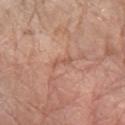{"biopsy_status": "not biopsied; imaged during a skin examination", "image": {"source": "total-body photography crop", "field_of_view_mm": 15}, "site": "left forearm", "automated_metrics": {"vs_skin_darker_L": 7.0, "vs_skin_contrast_norm": 5.0, "border_irregularity_0_10": 5.5, "nevus_likeness_0_100": 0, "lesion_detection_confidence_0_100": 90}, "patient": {"sex": "female", "age_approx": 75}, "lighting": "white-light"}